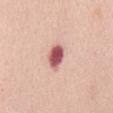No biopsy was performed on this lesion — it was imaged during a full skin examination and was not determined to be concerning. The subject is a female aged 63–67. The lesion is on the mid back. Automated image analysis of the tile measured an area of roughly 6 mm² and an eccentricity of roughly 0.8. The analysis additionally found a mean CIELAB color near L≈57 a*≈30 b*≈21, about 20 CIELAB-L* units darker than the surrounding skin, and a normalized border contrast of about 12. And it measured a color-variation rating of about 5/10 and a peripheral color-asymmetry measure near 1.5. A region of skin cropped from a whole-body photographic capture, roughly 15 mm wide. Imaged with white-light lighting. Longest diameter approximately 3.5 mm.